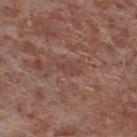Assessment: Part of a total-body skin-imaging series; this lesion was reviewed on a skin check and was not flagged for biopsy. Context: A male subject, approximately 55 years of age. Cropped from a total-body skin-imaging series; the visible field is about 15 mm. Located on the leg.